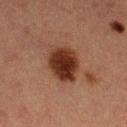workup: no biopsy performed (imaged during a skin exam) | size: ~4.5 mm (longest diameter) | illumination: cross-polarized illumination | anatomic site: the left thigh | image: total-body-photography crop, ~15 mm field of view | subject: female, aged around 40.